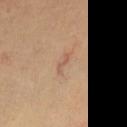Recorded during total-body skin imaging; not selected for excision or biopsy.
The total-body-photography lesion software estimated a lesion area of about 1.5 mm², an eccentricity of roughly 0.95, and a shape-asymmetry score of about 0.6 (0 = symmetric).
This is a cross-polarized tile.
A male subject aged 68–72.
Approximately 2.5 mm at its widest.
A close-up tile cropped from a whole-body skin photograph, about 15 mm across.
On the chest.